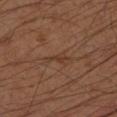Captured during whole-body skin photography for melanoma surveillance; the lesion was not biopsied.
The lesion is located on the left forearm.
Cropped from a whole-body photographic skin survey; the tile spans about 15 mm.
The patient is a male about 40 years old.